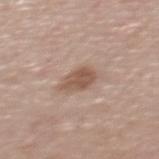Clinical impression: The lesion was tiled from a total-body skin photograph and was not biopsied. Image and clinical context: The lesion's longest dimension is about 3.5 mm. This image is a 15 mm lesion crop taken from a total-body photograph. The lesion is on the mid back. A female subject, aged 48 to 52. The tile uses white-light illumination. Automated tile analysis of the lesion measured a lesion area of about 6 mm², a shape eccentricity near 0.7, and a symmetry-axis asymmetry near 0.2. The analysis additionally found internal color variation of about 2.5 on a 0–10 scale and a peripheral color-asymmetry measure near 1. It also reported a nevus-likeness score of about 70/100 and a lesion-detection confidence of about 100/100.